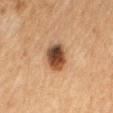Impression:
Part of a total-body skin-imaging series; this lesion was reviewed on a skin check and was not flagged for biopsy.
Clinical summary:
A 15 mm crop from a total-body photograph taken for skin-cancer surveillance. Captured under cross-polarized illumination. A female patient aged 58–62. An algorithmic analysis of the crop reported a border-irregularity rating of about 1.5/10. On the mid back.